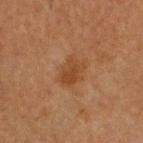follow-up: imaged on a skin check; not biopsied
subject: male, aged around 60
anatomic site: the head or neck
image: total-body-photography crop, ~15 mm field of view